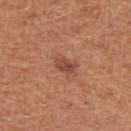Case summary:
- workup — total-body-photography surveillance lesion; no biopsy
- TBP lesion metrics — an area of roughly 4 mm², an eccentricity of roughly 0.75, and two-axis asymmetry of about 0.25; a within-lesion color-variation index near 3/10 and radial color variation of about 1.5
- body site — the left upper arm
- diameter — about 2.5 mm
- lighting — white-light illumination
- image — total-body-photography crop, ~15 mm field of view
- subject — female, approximately 40 years of age